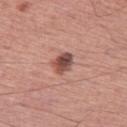Impression:
The lesion was tiled from a total-body skin photograph and was not biopsied.
Clinical summary:
The lesion is located on the left thigh. Longest diameter approximately 3 mm. Captured under white-light illumination. A close-up tile cropped from a whole-body skin photograph, about 15 mm across. A male patient in their 50s.